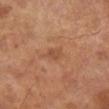| field | value |
|---|---|
| follow-up | total-body-photography surveillance lesion; no biopsy |
| image source | total-body-photography crop, ~15 mm field of view |
| diameter | ≈3 mm |
| tile lighting | cross-polarized illumination |
| patient | male, in their mid- to late 60s |
| anatomic site | the left lower leg |